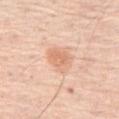Captured during whole-body skin photography for melanoma surveillance; the lesion was not biopsied. The lesion is on the right thigh. A 15 mm crop from a total-body photograph taken for skin-cancer surveillance. A male patient, aged 78–82.